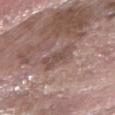Clinical summary: The patient is a male aged 58 to 62. Captured under white-light illumination. The lesion is located on the right forearm. Cropped from a total-body skin-imaging series; the visible field is about 15 mm.